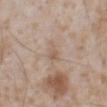Impression: No biopsy was performed on this lesion — it was imaged during a full skin examination and was not determined to be concerning. Image and clinical context: An algorithmic analysis of the crop reported an area of roughly 4.5 mm², a shape eccentricity near 0.8, and a shape-asymmetry score of about 0.3 (0 = symmetric). It also reported roughly 6 lightness units darker than nearby skin and a normalized border contrast of about 4.5. The software also gave a nevus-likeness score of about 0/100 and a lesion-detection confidence of about 100/100. Cropped from a total-body skin-imaging series; the visible field is about 15 mm. About 3 mm across. A male patient aged 63–67. Captured under white-light illumination. From the abdomen.